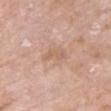  biopsy_status: not biopsied; imaged during a skin examination
  patient:
    sex: female
    age_approx: 75
  image:
    source: total-body photography crop
    field_of_view_mm: 15
  lighting: white-light
  lesion_size:
    long_diameter_mm_approx: 2.5
  site: chest